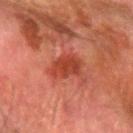The patient is a male in their 80s. Cropped from a whole-body photographic skin survey; the tile spans about 15 mm. Located on the arm. The lesion-visualizer software estimated a shape-asymmetry score of about 0.25 (0 = symmetric). And it measured a lesion color around L≈34 a*≈28 b*≈28 in CIELAB, about 8 CIELAB-L* units darker than the surrounding skin, and a normalized lesion–skin contrast near 7.5. The software also gave a border-irregularity rating of about 2.5/10, internal color variation of about 3 on a 0–10 scale, and a peripheral color-asymmetry measure near 1. It also reported an automated nevus-likeness rating near 5 out of 100 and a lesion-detection confidence of about 100/100.